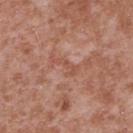This lesion was catalogued during total-body skin photography and was not selected for biopsy. Imaged with white-light lighting. The lesion is located on the upper back. A male subject, aged 43–47. A region of skin cropped from a whole-body photographic capture, roughly 15 mm wide.